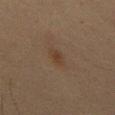biopsy_status: not biopsied; imaged during a skin examination
lesion_size:
  long_diameter_mm_approx: 2.5
site: chest
image:
  source: total-body photography crop
  field_of_view_mm: 15
patient:
  sex: male
  age_approx: 65